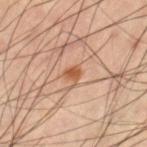Q: Was a biopsy performed?
A: catalogued during a skin exam; not biopsied
Q: What kind of image is this?
A: 15 mm crop, total-body photography
Q: Lesion size?
A: about 2 mm
Q: Where on the body is the lesion?
A: the left thigh
Q: Who is the patient?
A: male, aged 58–62
Q: What lighting was used for the tile?
A: cross-polarized